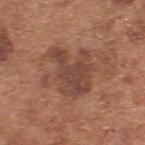workup = catalogued during a skin exam; not biopsied | diameter = about 5.5 mm | imaging modality = 15 mm crop, total-body photography | image-analysis metrics = a lesion area of about 17 mm², a shape eccentricity near 0.6, and two-axis asymmetry of about 0.4; an average lesion color of about L≈44 a*≈21 b*≈27 (CIELAB) and roughly 9 lightness units darker than nearby skin | anatomic site = the upper back | tile lighting = white-light illumination | patient = male, in their mid- to late 60s.